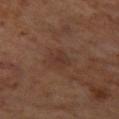On the leg. A male patient, aged 58 to 62. Automated tile analysis of the lesion measured an average lesion color of about L≈31 a*≈18 b*≈23 (CIELAB) and about 5 CIELAB-L* units darker than the surrounding skin. It also reported a nevus-likeness score of about 0/100 and a detector confidence of about 100 out of 100 that the crop contains a lesion. A 15 mm close-up tile from a total-body photography series done for melanoma screening. The tile uses cross-polarized illumination. The recorded lesion diameter is about 3 mm.